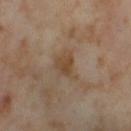{"biopsy_status": "not biopsied; imaged during a skin examination", "patient": {"sex": "female", "age_approx": 55}, "site": "right thigh", "automated_metrics": {"cielab_L": 46, "cielab_a": 15, "cielab_b": 30, "vs_skin_darker_L": 8.0, "vs_skin_contrast_norm": 7.0, "border_irregularity_0_10": 4.0, "color_variation_0_10": 3.0, "peripheral_color_asymmetry": 1.0, "nevus_likeness_0_100": 0, "lesion_detection_confidence_0_100": 100}, "lighting": "cross-polarized", "lesion_size": {"long_diameter_mm_approx": 4.0}, "image": {"source": "total-body photography crop", "field_of_view_mm": 15}}